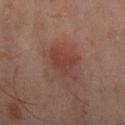workup: no biopsy performed (imaged during a skin exam)
TBP lesion metrics: a footprint of about 7.5 mm², an outline eccentricity of about 0.55 (0 = round, 1 = elongated), and a symmetry-axis asymmetry near 0.35; a lesion-detection confidence of about 100/100
acquisition: 15 mm crop, total-body photography
size: ~3.5 mm (longest diameter)
body site: the right lower leg
tile lighting: cross-polarized illumination
subject: male, in their mid-40s